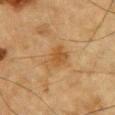This lesion was catalogued during total-body skin photography and was not selected for biopsy. A close-up tile cropped from a whole-body skin photograph, about 15 mm across. A male patient, aged 83 or older. Captured under cross-polarized illumination. About 3.5 mm across. The lesion is on the chest. Automated image analysis of the tile measured a footprint of about 6.5 mm² and a shape-asymmetry score of about 0.35 (0 = symmetric). The analysis additionally found a mean CIELAB color near L≈46 a*≈18 b*≈37 and roughly 7 lightness units darker than nearby skin.